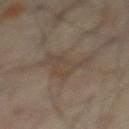{"biopsy_status": "not biopsied; imaged during a skin examination", "image": {"source": "total-body photography crop", "field_of_view_mm": 15}, "site": "front of the torso", "patient": {"sex": "male", "age_approx": 65}}